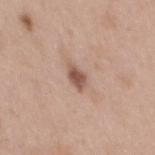notes: catalogued during a skin exam; not biopsied
patient: female, aged approximately 40
location: the mid back
imaging modality: 15 mm crop, total-body photography
automated metrics: a classifier nevus-likeness of about 85/100 and lesion-presence confidence of about 100/100